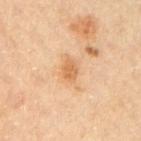Assessment: Recorded during total-body skin imaging; not selected for excision or biopsy. Context: Located on the right upper arm. A 15 mm close-up extracted from a 3D total-body photography capture. Captured under cross-polarized illumination. The total-body-photography lesion software estimated an average lesion color of about L≈61 a*≈21 b*≈39 (CIELAB), a lesion–skin lightness drop of about 9, and a normalized lesion–skin contrast near 7. The analysis additionally found a border-irregularity rating of about 1.5/10 and a peripheral color-asymmetry measure near 0.5. And it measured a classifier nevus-likeness of about 0/100. A male patient aged 58 to 62. Longest diameter approximately 2.5 mm.